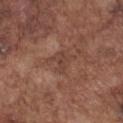The lesion was photographed on a routine skin check and not biopsied; there is no pathology result. Cropped from a total-body skin-imaging series; the visible field is about 15 mm. A male patient aged 73 to 77. From the front of the torso. Longest diameter approximately 3.5 mm.